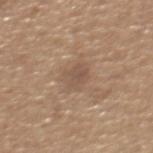Imaged during a routine full-body skin examination; the lesion was not biopsied and no histopathology is available. Automated tile analysis of the lesion measured a lesion color around L≈52 a*≈16 b*≈28 in CIELAB, roughly 7 lightness units darker than nearby skin, and a normalized lesion–skin contrast near 5. It also reported radial color variation of about 0.5. The software also gave a classifier nevus-likeness of about 0/100 and a lesion-detection confidence of about 100/100. On the upper back. The lesion's longest dimension is about 3 mm. The subject is a male roughly 65 years of age. A region of skin cropped from a whole-body photographic capture, roughly 15 mm wide. The tile uses white-light illumination.